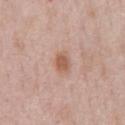Q: What is the imaging modality?
A: 15 mm crop, total-body photography
Q: How large is the lesion?
A: ≈2.5 mm
Q: What did automated image analysis measure?
A: an eccentricity of roughly 0.7 and a shape-asymmetry score of about 0.2 (0 = symmetric); a border-irregularity rating of about 1.5/10, internal color variation of about 2 on a 0–10 scale, and a peripheral color-asymmetry measure near 0.5
Q: Who is the patient?
A: male, roughly 50 years of age
Q: Where on the body is the lesion?
A: the chest